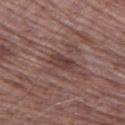The lesion was photographed on a routine skin check and not biopsied; there is no pathology result.
The subject is a male about 70 years old.
A close-up tile cropped from a whole-body skin photograph, about 15 mm across.
Automated image analysis of the tile measured a lesion color around L≈39 a*≈20 b*≈20 in CIELAB and a normalized border contrast of about 7.
On the left thigh.
Measured at roughly 3.5 mm in maximum diameter.